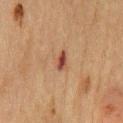workup: imaged on a skin check; not biopsied
subject: male, aged 73–77
tile lighting: cross-polarized
anatomic site: the chest
acquisition: 15 mm crop, total-body photography
diameter: ≈2.5 mm